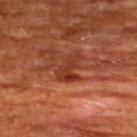Clinical impression:
Imaged during a routine full-body skin examination; the lesion was not biopsied and no histopathology is available.
Context:
A 15 mm close-up extracted from a 3D total-body photography capture. From the upper back. The lesion's longest dimension is about 3.5 mm. This is a cross-polarized tile. The subject is a male approximately 65 years of age.